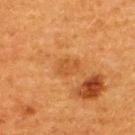This lesion was catalogued during total-body skin photography and was not selected for biopsy. The lesion-visualizer software estimated an area of roughly 3 mm², a shape eccentricity near 0.75, and a shape-asymmetry score of about 0.5 (0 = symmetric). The software also gave a border-irregularity index near 5.5/10 and a within-lesion color-variation index near 0.5/10. A lesion tile, about 15 mm wide, cut from a 3D total-body photograph. Approximately 2.5 mm at its widest. Captured under cross-polarized illumination. Located on the upper back. The subject is a female about 40 years old.